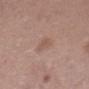Recorded during total-body skin imaging; not selected for excision or biopsy.
A 15 mm crop from a total-body photograph taken for skin-cancer surveillance.
From the abdomen.
A female subject roughly 40 years of age.